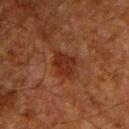{"biopsy_status": "not biopsied; imaged during a skin examination", "patient": {"sex": "male", "age_approx": 60}, "site": "upper back", "automated_metrics": {"nevus_likeness_0_100": 30, "lesion_detection_confidence_0_100": 100}, "image": {"source": "total-body photography crop", "field_of_view_mm": 15}, "lighting": "cross-polarized", "lesion_size": {"long_diameter_mm_approx": 3.5}}